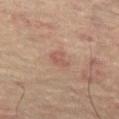Acquisition and patient details:
A male patient, roughly 60 years of age. An algorithmic analysis of the crop reported border irregularity of about 2.5 on a 0–10 scale, a color-variation rating of about 1.5/10, and peripheral color asymmetry of about 0.5. And it measured an automated nevus-likeness rating near 10 out of 100 and a detector confidence of about 100 out of 100 that the crop contains a lesion. The tile uses cross-polarized illumination. A 15 mm close-up extracted from a 3D total-body photography capture. Located on the left thigh. Approximately 2.5 mm at its widest.